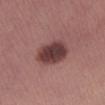Clinical impression:
This lesion was catalogued during total-body skin photography and was not selected for biopsy.
Image and clinical context:
About 5 mm across. Located on the leg. A 15 mm close-up extracted from a 3D total-body photography capture. Imaged with white-light lighting. The subject is a female aged around 25. Automated image analysis of the tile measured an average lesion color of about L≈39 a*≈21 b*≈19 (CIELAB) and a lesion-to-skin contrast of about 11.5 (normalized; higher = more distinct).